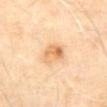| key | value |
|---|---|
| follow-up | imaged on a skin check; not biopsied |